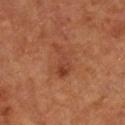The lesion was tiled from a total-body skin photograph and was not biopsied. This image is a 15 mm lesion crop taken from a total-body photograph. Located on the right lower leg. Longest diameter approximately 4 mm. A male subject aged 63–67.